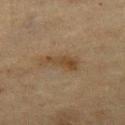notes: catalogued during a skin exam; not biopsied | subject: female, aged 53 to 57 | image-analysis metrics: a lesion area of about 7.5 mm² and two-axis asymmetry of about 0.35; border irregularity of about 4.5 on a 0–10 scale, internal color variation of about 3 on a 0–10 scale, and a peripheral color-asymmetry measure near 1; a classifier nevus-likeness of about 5/100 and a detector confidence of about 100 out of 100 that the crop contains a lesion | illumination: cross-polarized illumination | body site: the left thigh | imaging modality: total-body-photography crop, ~15 mm field of view | lesion size: about 4.5 mm.